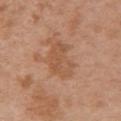Impression: Captured during whole-body skin photography for melanoma surveillance; the lesion was not biopsied. Acquisition and patient details: The tile uses white-light illumination. A female subject approximately 65 years of age. The recorded lesion diameter is about 4.5 mm. A region of skin cropped from a whole-body photographic capture, roughly 15 mm wide. The lesion is on the front of the torso.